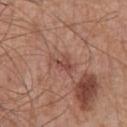Impression: The lesion was tiled from a total-body skin photograph and was not biopsied. Context: A roughly 15 mm field-of-view crop from a total-body skin photograph. The lesion is located on the front of the torso. The tile uses white-light illumination. The subject is a male in their mid-60s.